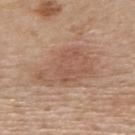Assessment: Part of a total-body skin-imaging series; this lesion was reviewed on a skin check and was not flagged for biopsy. Image and clinical context: An algorithmic analysis of the crop reported an area of roughly 26 mm², a shape eccentricity near 0.8, and a symmetry-axis asymmetry near 0.3. And it measured a border-irregularity rating of about 3.5/10 and radial color variation of about 1. The analysis additionally found a nevus-likeness score of about 5/100 and a detector confidence of about 100 out of 100 that the crop contains a lesion. On the upper back. About 7.5 mm across. Captured under white-light illumination. The patient is a male in their 70s. Cropped from a whole-body photographic skin survey; the tile spans about 15 mm.